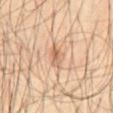The lesion was tiled from a total-body skin photograph and was not biopsied. The lesion's longest dimension is about 3 mm. A close-up tile cropped from a whole-body skin photograph, about 15 mm across. The lesion is on the abdomen. A subject in their mid- to late 60s. Imaged with cross-polarized lighting.